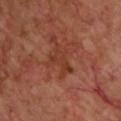| field | value |
|---|---|
| imaging modality | total-body-photography crop, ~15 mm field of view |
| site | the chest |
| diameter | about 4 mm |
| subject | male, aged 68 to 72 |